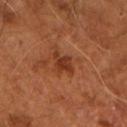notes: catalogued during a skin exam; not biopsied | illumination: cross-polarized | TBP lesion metrics: an average lesion color of about L≈35 a*≈26 b*≈34 (CIELAB) and a normalized lesion–skin contrast near 8; a border-irregularity rating of about 4/10 | subject: male, approximately 65 years of age | diameter: about 3 mm | acquisition: total-body-photography crop, ~15 mm field of view.